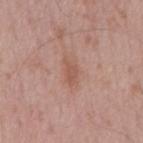The lesion was photographed on a routine skin check and not biopsied; there is no pathology result.
A 15 mm crop from a total-body photograph taken for skin-cancer surveillance.
The tile uses white-light illumination.
The patient is a male approximately 55 years of age.
The lesion is on the mid back.